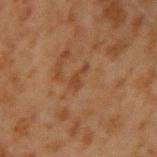<record>
  <biopsy_status>not biopsied; imaged during a skin examination</biopsy_status>
  <image>
    <source>total-body photography crop</source>
    <field_of_view_mm>15</field_of_view_mm>
  </image>
  <lesion_size>
    <long_diameter_mm_approx>3.0</long_diameter_mm_approx>
  </lesion_size>
  <patient>
    <sex>male</sex>
    <age_approx>45</age_approx>
  </patient>
  <site>right upper arm</site>
</record>